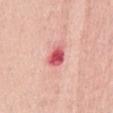Findings:
– notes — catalogued during a skin exam; not biopsied
– site — the back
– patient — female, aged 58 to 62
– image source — 15 mm crop, total-body photography